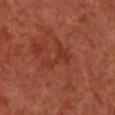Recorded during total-body skin imaging; not selected for excision or biopsy.
Longest diameter approximately 4 mm.
A female patient, roughly 50 years of age.
The tile uses cross-polarized illumination.
The lesion is located on the chest.
A region of skin cropped from a whole-body photographic capture, roughly 15 mm wide.